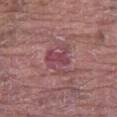– notes: imaged on a skin check; not biopsied
– TBP lesion metrics: a shape eccentricity near 0.5 and a symmetry-axis asymmetry near 0.4; a lesion color around L≈45 a*≈27 b*≈16 in CIELAB, about 8 CIELAB-L* units darker than the surrounding skin, and a normalized lesion–skin contrast near 6.5
– site: the right thigh
– patient: male, in their 80s
– tile lighting: white-light
– diameter: about 4 mm
– imaging modality: 15 mm crop, total-body photography